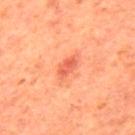<lesion>
<biopsy_status>not biopsied; imaged during a skin examination</biopsy_status>
<image>
  <source>total-body photography crop</source>
  <field_of_view_mm>15</field_of_view_mm>
</image>
<patient>
  <sex>male</sex>
  <age_approx>65</age_approx>
</patient>
<site>mid back</site>
<lesion_size>
  <long_diameter_mm_approx>3.0</long_diameter_mm_approx>
</lesion_size>
<lighting>cross-polarized</lighting>
</lesion>